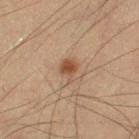biopsy status: catalogued during a skin exam; not biopsied | lesion size: ~2.5 mm (longest diameter) | patient: male, roughly 55 years of age | image source: total-body-photography crop, ~15 mm field of view | lighting: cross-polarized illumination | anatomic site: the left thigh.